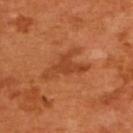biopsy_status: not biopsied; imaged during a skin examination